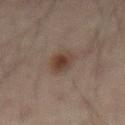– notes: imaged on a skin check; not biopsied
– lighting: cross-polarized illumination
– patient: male, approximately 45 years of age
– diameter: ≈3 mm
– site: the abdomen
– acquisition: 15 mm crop, total-body photography